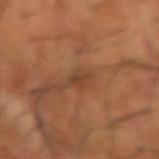Case summary:
• follow-up — no biopsy performed (imaged during a skin exam)
• TBP lesion metrics — a lesion color around L≈41 a*≈22 b*≈31 in CIELAB and a lesion–skin lightness drop of about 7; a nevus-likeness score of about 0/100 and a lesion-detection confidence of about 85/100
• site — the left forearm
• image — ~15 mm crop, total-body skin-cancer survey
• illumination — cross-polarized
• subject — male, roughly 65 years of age